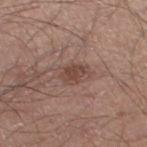Findings:
- biopsy status — catalogued during a skin exam; not biopsied
- diameter — ≈4 mm
- subject — male, roughly 50 years of age
- illumination — white-light illumination
- imaging modality — 15 mm crop, total-body photography
- location — the leg